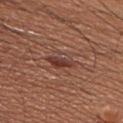A male subject aged approximately 60. The lesion is on the chest. A 15 mm close-up extracted from a 3D total-body photography capture.